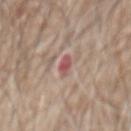<case>
  <biopsy_status>not biopsied; imaged during a skin examination</biopsy_status>
  <image>
    <source>total-body photography crop</source>
    <field_of_view_mm>15</field_of_view_mm>
  </image>
  <patient>
    <sex>male</sex>
    <age_approx>80</age_approx>
  </patient>
  <lesion_size>
    <long_diameter_mm_approx>3.0</long_diameter_mm_approx>
  </lesion_size>
  <site>abdomen</site>
  <lighting>white-light</lighting>
  <automated_metrics>
    <area_mm2_approx>2.5</area_mm2_approx>
    <eccentricity>0.95</eccentricity>
    <shape_asymmetry>0.35</shape_asymmetry>
    <border_irregularity_0_10>3.5</border_irregularity_0_10>
    <peripheral_color_asymmetry>0.0</peripheral_color_asymmetry>
  </automated_metrics>
</case>